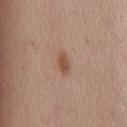biopsy status: total-body-photography surveillance lesion; no biopsy
body site: the front of the torso
lesion size: ~2.5 mm (longest diameter)
image source: ~15 mm tile from a whole-body skin photo
automated metrics: a footprint of about 4 mm² and an outline eccentricity of about 0.8 (0 = round, 1 = elongated); a nevus-likeness score of about 90/100 and a detector confidence of about 100 out of 100 that the crop contains a lesion
patient: female, aged approximately 45
illumination: white-light illumination